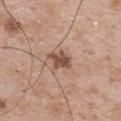Context: The lesion is on the back. About 3 mm across. A male subject, approximately 50 years of age. This is a white-light tile. A 15 mm close-up tile from a total-body photography series done for melanoma screening.